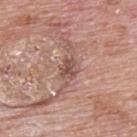Q: Was this lesion biopsied?
A: total-body-photography surveillance lesion; no biopsy
Q: What are the patient's age and sex?
A: male, aged 68 to 72
Q: What is the imaging modality?
A: ~15 mm crop, total-body skin-cancer survey
Q: What is the anatomic site?
A: the upper back
Q: Automated lesion metrics?
A: an area of roughly 4 mm², a shape eccentricity near 0.7, and a shape-asymmetry score of about 0.2 (0 = symmetric); border irregularity of about 2 on a 0–10 scale, a color-variation rating of about 2/10, and peripheral color asymmetry of about 1; an automated nevus-likeness rating near 0 out of 100 and a detector confidence of about 90 out of 100 that the crop contains a lesion
Q: Illumination type?
A: white-light